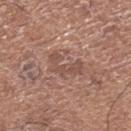Case summary:
– follow-up — no biopsy performed (imaged during a skin exam)
– lesion size — about 4 mm
– anatomic site — the right lower leg
– subject — female, approximately 50 years of age
– automated metrics — about 8 CIELAB-L* units darker than the surrounding skin and a normalized border contrast of about 6; an automated nevus-likeness rating near 0 out of 100
– lighting — white-light
– image source — ~15 mm crop, total-body skin-cancer survey